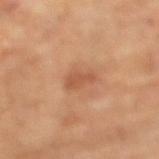Captured during whole-body skin photography for melanoma surveillance; the lesion was not biopsied.
The total-body-photography lesion software estimated a nevus-likeness score of about 5/100 and lesion-presence confidence of about 100/100.
The lesion's longest dimension is about 3 mm.
On the right lower leg.
A region of skin cropped from a whole-body photographic capture, roughly 15 mm wide.
A female patient in their 70s.
The tile uses cross-polarized illumination.